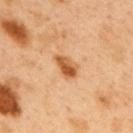Clinical impression: The lesion was photographed on a routine skin check and not biopsied; there is no pathology result. Image and clinical context: Captured under cross-polarized illumination. The patient is a male aged around 50. The lesion's longest dimension is about 3.5 mm. The lesion is located on the upper back. Cropped from a whole-body photographic skin survey; the tile spans about 15 mm.